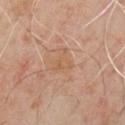Assessment:
No biopsy was performed on this lesion — it was imaged during a full skin examination and was not determined to be concerning.
Acquisition and patient details:
Longest diameter approximately 3.5 mm. On the front of the torso. The total-body-photography lesion software estimated a lesion area of about 4.5 mm², an eccentricity of roughly 0.75, and a shape-asymmetry score of about 0.55 (0 = symmetric). It also reported a nevus-likeness score of about 0/100. Captured under cross-polarized illumination. A close-up tile cropped from a whole-body skin photograph, about 15 mm across. The patient is a male in their 50s.